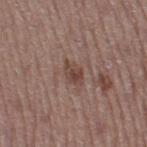workup: no biopsy performed (imaged during a skin exam) | subject: female, aged approximately 50 | site: the right thigh | image source: ~15 mm tile from a whole-body skin photo.